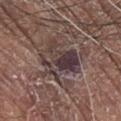Conclusion: On excision, pathology confirmed an invasive squamous cell carcinoma, classified as a malignant lesion.